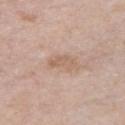A female subject approximately 85 years of age or older. Cropped from a total-body skin-imaging series; the visible field is about 15 mm. The lesion's longest dimension is about 3.5 mm. From the chest. The tile uses white-light illumination.